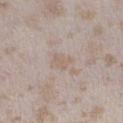Assessment: Part of a total-body skin-imaging series; this lesion was reviewed on a skin check and was not flagged for biopsy. Acquisition and patient details: From the left lower leg. Measured at roughly 2.5 mm in maximum diameter. A female subject, in their mid- to late 20s. Cropped from a total-body skin-imaging series; the visible field is about 15 mm. This is a white-light tile.